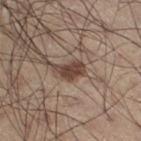Clinical impression:
Recorded during total-body skin imaging; not selected for excision or biopsy.